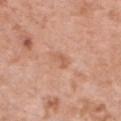follow-up: no biopsy performed (imaged during a skin exam)
patient: female, roughly 50 years of age
anatomic site: the chest
TBP lesion metrics: a border-irregularity index near 2.5/10, a within-lesion color-variation index near 1.5/10, and radial color variation of about 0.5
image: ~15 mm crop, total-body skin-cancer survey
size: ≈2.5 mm